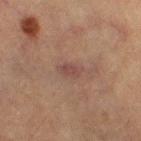No biopsy was performed on this lesion — it was imaged during a full skin examination and was not determined to be concerning. This is a cross-polarized tile. A female patient, roughly 60 years of age. Measured at roughly 2.5 mm in maximum diameter. Cropped from a total-body skin-imaging series; the visible field is about 15 mm. On the leg.